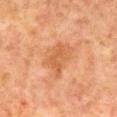This lesion was catalogued during total-body skin photography and was not selected for biopsy. The tile uses cross-polarized illumination. A male patient, approximately 60 years of age. The lesion's longest dimension is about 4.5 mm. Located on the mid back. A lesion tile, about 15 mm wide, cut from a 3D total-body photograph.